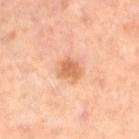workup — catalogued during a skin exam; not biopsied | image — ~15 mm tile from a whole-body skin photo | subject — female, aged 48–52 | location — the lower back.